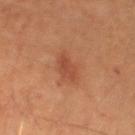Case summary:
* image-analysis metrics: a shape eccentricity near 0.9 and a symmetry-axis asymmetry near 0.3; a lesion color around L≈42 a*≈25 b*≈31 in CIELAB, a lesion–skin lightness drop of about 7, and a normalized border contrast of about 6
* image: total-body-photography crop, ~15 mm field of view
* site: the right upper arm
* diameter: ≈3.5 mm
* tile lighting: cross-polarized illumination
* patient: male, in their mid-60s